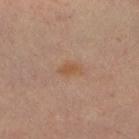| field | value |
|---|---|
| notes | imaged on a skin check; not biopsied |
| site | the left leg |
| patient | female, roughly 40 years of age |
| acquisition | total-body-photography crop, ~15 mm field of view |
| diameter | ≈2.5 mm |